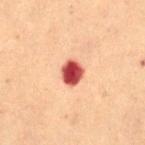Findings:
- image-analysis metrics: a lesion area of about 5.5 mm², an eccentricity of roughly 0.55, and a symmetry-axis asymmetry near 0.2; an average lesion color of about L≈39 a*≈31 b*≈25 (CIELAB), roughly 20 lightness units darker than nearby skin, and a normalized lesion–skin contrast near 15
- site: the lower back
- size: ≈3 mm
- tile lighting: cross-polarized illumination
- acquisition: ~15 mm tile from a whole-body skin photo
- patient: female, aged approximately 80